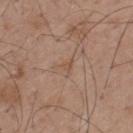biopsy status — no biopsy performed (imaged during a skin exam) | lighting — white-light illumination | acquisition — total-body-photography crop, ~15 mm field of view | patient — male, aged around 50 | TBP lesion metrics — a mean CIELAB color near L≈52 a*≈17 b*≈29, a lesion–skin lightness drop of about 6, and a lesion-to-skin contrast of about 5 (normalized; higher = more distinct); border irregularity of about 6 on a 0–10 scale, a color-variation rating of about 0.5/10, and radial color variation of about 0 | site — the upper back.